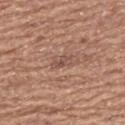The lesion was photographed on a routine skin check and not biopsied; there is no pathology result. Located on the upper back. The subject is a female aged around 65. The lesion's longest dimension is about 2.5 mm. A roughly 15 mm field-of-view crop from a total-body skin photograph. Imaged with white-light lighting.